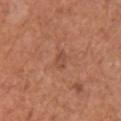Captured during whole-body skin photography for melanoma surveillance; the lesion was not biopsied. Cropped from a whole-body photographic skin survey; the tile spans about 15 mm. Approximately 2.5 mm at its widest. The tile uses white-light illumination. The lesion-visualizer software estimated an area of roughly 3.5 mm², an outline eccentricity of about 0.8 (0 = round, 1 = elongated), and two-axis asymmetry of about 0.2. The software also gave a border-irregularity rating of about 2/10, a within-lesion color-variation index near 2/10, and a peripheral color-asymmetry measure near 0.5. A female subject, about 50 years old. From the right upper arm.